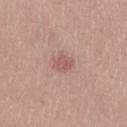Imaged during a routine full-body skin examination; the lesion was not biopsied and no histopathology is available.
A 15 mm close-up tile from a total-body photography series done for melanoma screening.
Imaged with white-light lighting.
A female subject aged 18–22.
The lesion is located on the left lower leg.
Measured at roughly 3 mm in maximum diameter.
Automated tile analysis of the lesion measured about 8 CIELAB-L* units darker than the surrounding skin and a normalized lesion–skin contrast near 5.5. And it measured a border-irregularity index near 3/10. And it measured a nevus-likeness score of about 0/100 and a lesion-detection confidence of about 100/100.